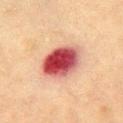Impression: The lesion was tiled from a total-body skin photograph and was not biopsied. Background: The lesion is on the right upper arm. The lesion's longest dimension is about 5 mm. A 15 mm close-up extracted from a 3D total-body photography capture. A male patient aged 73 to 77. The tile uses cross-polarized illumination.